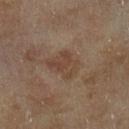This lesion was catalogued during total-body skin photography and was not selected for biopsy. A close-up tile cropped from a whole-body skin photograph, about 15 mm across. On the leg. The subject is a female approximately 60 years of age.